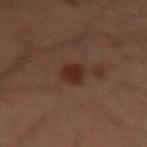Findings:
* biopsy status — no biopsy performed (imaged during a skin exam)
* anatomic site — the back
* subject — male, aged 58 to 62
* lighting — cross-polarized illumination
* acquisition — 15 mm crop, total-body photography